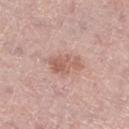Clinical impression: Recorded during total-body skin imaging; not selected for excision or biopsy. Image and clinical context: The lesion's longest dimension is about 4 mm. Automated image analysis of the tile measured a mean CIELAB color near L≈59 a*≈22 b*≈26, roughly 9 lightness units darker than nearby skin, and a normalized border contrast of about 6.5. And it measured a nevus-likeness score of about 30/100 and lesion-presence confidence of about 100/100. A female subject approximately 65 years of age. On the left lower leg. A 15 mm close-up extracted from a 3D total-body photography capture. This is a white-light tile.